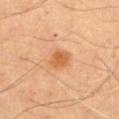This lesion was catalogued during total-body skin photography and was not selected for biopsy.